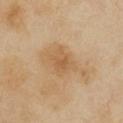The tile uses cross-polarized illumination. The lesion is located on the chest. A close-up tile cropped from a whole-body skin photograph, about 15 mm across. Longest diameter approximately 3 mm. A female patient, approximately 40 years of age.